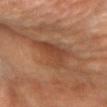{
  "biopsy_status": "not biopsied; imaged during a skin examination",
  "site": "right forearm",
  "image": {
    "source": "total-body photography crop",
    "field_of_view_mm": 15
  },
  "patient": {
    "sex": "female",
    "age_approx": 70
  }
}